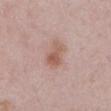| field | value |
|---|---|
| workup | total-body-photography surveillance lesion; no biopsy |
| site | the left upper arm |
| image | total-body-photography crop, ~15 mm field of view |
| patient | male, aged 48–52 |
| diameter | ~4 mm (longest diameter) |
| lighting | white-light |
| image-analysis metrics | two-axis asymmetry of about 0.3; a mean CIELAB color near L≈57 a*≈20 b*≈26, a lesion–skin lightness drop of about 9, and a normalized lesion–skin contrast near 7; a border-irregularity index near 3.5/10 and a color-variation rating of about 3.5/10 |